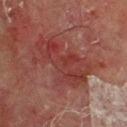Captured under cross-polarized illumination. Longest diameter approximately 7.5 mm. Automated image analysis of the tile measured a mean CIELAB color near L≈31 a*≈24 b*≈21, about 6 CIELAB-L* units darker than the surrounding skin, and a normalized border contrast of about 6.5. It also reported a border-irregularity index near 6/10, a within-lesion color-variation index near 4.5/10, and a peripheral color-asymmetry measure near 1.5. The software also gave a nevus-likeness score of about 0/100 and lesion-presence confidence of about 90/100. A close-up tile cropped from a whole-body skin photograph, about 15 mm across. A male patient aged approximately 70. Located on the chest.